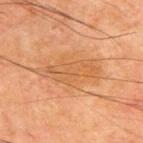The lesion's longest dimension is about 7 mm. The lesion is on the upper back. The tile uses cross-polarized illumination. An algorithmic analysis of the crop reported an area of roughly 15 mm², an eccentricity of roughly 0.9, and two-axis asymmetry of about 0.25. And it measured a lesion color around L≈47 a*≈20 b*≈34 in CIELAB and about 6 CIELAB-L* units darker than the surrounding skin. The analysis additionally found a border-irregularity index near 4/10, a within-lesion color-variation index near 3/10, and peripheral color asymmetry of about 1. And it measured lesion-presence confidence of about 100/100. The subject is a male in their mid- to late 60s. A lesion tile, about 15 mm wide, cut from a 3D total-body photograph.